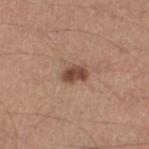workup: imaged on a skin check; not biopsied | body site: the left lower leg | image source: ~15 mm crop, total-body skin-cancer survey | automated metrics: an area of roughly 5 mm², an outline eccentricity of about 0.75 (0 = round, 1 = elongated), and two-axis asymmetry of about 0.3; a lesion color around L≈47 a*≈21 b*≈27 in CIELAB and a normalized lesion–skin contrast near 9.5; a within-lesion color-variation index near 3/10 | diameter: ~3 mm (longest diameter) | patient: male, roughly 55 years of age.